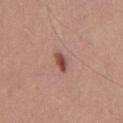This lesion was catalogued during total-body skin photography and was not selected for biopsy. A male subject, approximately 55 years of age. This is a white-light tile. Automated tile analysis of the lesion measured a lesion area of about 3.5 mm² and a symmetry-axis asymmetry near 0.3. The analysis additionally found a lesion color around L≈49 a*≈22 b*≈27 in CIELAB, a lesion–skin lightness drop of about 13, and a normalized lesion–skin contrast near 9. The analysis additionally found a nevus-likeness score of about 100/100. Located on the chest. The lesion's longest dimension is about 2.5 mm. A close-up tile cropped from a whole-body skin photograph, about 15 mm across.